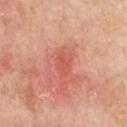Assessment:
No biopsy was performed on this lesion — it was imaged during a full skin examination and was not determined to be concerning.
Background:
The patient is a female in their 70s. The total-body-photography lesion software estimated a footprint of about 5 mm², an eccentricity of roughly 0.8, and a symmetry-axis asymmetry near 0.25. It also reported a border-irregularity rating of about 2.5/10 and radial color variation of about 0.5. The software also gave a classifier nevus-likeness of about 0/100 and lesion-presence confidence of about 100/100. The lesion is located on the front of the torso. A roughly 15 mm field-of-view crop from a total-body skin photograph. This is a white-light tile.